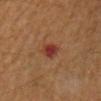{
  "biopsy_status": "not biopsied; imaged during a skin examination",
  "patient": {
    "sex": "male",
    "age_approx": 55
  },
  "automated_metrics": {
    "area_mm2_approx": 4.5,
    "eccentricity": 0.55,
    "shape_asymmetry": 0.25,
    "cielab_L": 32,
    "cielab_a": 25,
    "cielab_b": 25,
    "vs_skin_darker_L": 9.0,
    "vs_skin_contrast_norm": 9.0,
    "nevus_likeness_0_100": 0
  },
  "site": "left arm",
  "image": {
    "source": "total-body photography crop",
    "field_of_view_mm": 15
  }
}